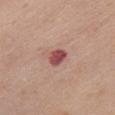{"biopsy_status": "not biopsied; imaged during a skin examination"}